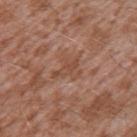notes = imaged on a skin check; not biopsied | image source = ~15 mm tile from a whole-body skin photo | anatomic site = the left upper arm | subject = male, in their mid-40s.